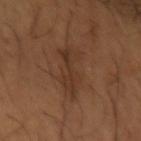Imaged during a routine full-body skin examination; the lesion was not biopsied and no histopathology is available. Imaged with cross-polarized lighting. Measured at roughly 6 mm in maximum diameter. A 15 mm crop from a total-body photograph taken for skin-cancer surveillance. The lesion is located on the left forearm. A male patient, aged around 65.